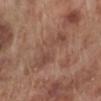anatomic site: the left lower leg | lesion size: ~6 mm (longest diameter) | tile lighting: white-light | acquisition: ~15 mm tile from a whole-body skin photo | automated metrics: an average lesion color of about L≈47 a*≈20 b*≈25 (CIELAB) and a normalized border contrast of about 5; a border-irregularity index near 7/10, a color-variation rating of about 2.5/10, and a peripheral color-asymmetry measure near 0.5 | patient: male, about 70 years old.